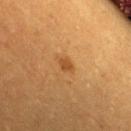Impression:
Part of a total-body skin-imaging series; this lesion was reviewed on a skin check and was not flagged for biopsy.
Context:
Longest diameter approximately 2 mm. From the chest. A 15 mm close-up tile from a total-body photography series done for melanoma screening. A female subject in their 30s.